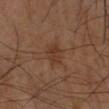{
  "biopsy_status": "not biopsied; imaged during a skin examination",
  "image": {
    "source": "total-body photography crop",
    "field_of_view_mm": 15
  },
  "lighting": "cross-polarized",
  "patient": {
    "sex": "male",
    "age_approx": 60
  },
  "site": "right arm"
}